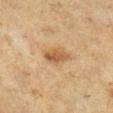subject = female, aged 53–57
anatomic site = the right lower leg
image source = ~15 mm tile from a whole-body skin photo